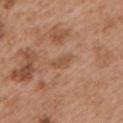Assessment:
Recorded during total-body skin imaging; not selected for excision or biopsy.
Image and clinical context:
Cropped from a total-body skin-imaging series; the visible field is about 15 mm. Located on the left upper arm. A male subject aged 53–57. This is a white-light tile. The total-body-photography lesion software estimated a footprint of about 2.5 mm², a shape eccentricity near 0.9, and a shape-asymmetry score of about 0.25 (0 = symmetric). The analysis additionally found a normalized lesion–skin contrast near 6. It also reported border irregularity of about 2.5 on a 0–10 scale and a peripheral color-asymmetry measure near 0. And it measured a nevus-likeness score of about 0/100.